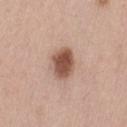Acquisition and patient details:
A close-up tile cropped from a whole-body skin photograph, about 15 mm across. Measured at roughly 3.5 mm in maximum diameter. This is a white-light tile. The lesion is on the leg. A female subject, aged 63–67.